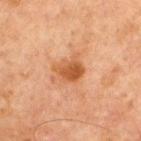Impression:
Part of a total-body skin-imaging series; this lesion was reviewed on a skin check and was not flagged for biopsy.
Acquisition and patient details:
The tile uses cross-polarized illumination. From the chest. Measured at roughly 3 mm in maximum diameter. Cropped from a total-body skin-imaging series; the visible field is about 15 mm. The patient is a male approximately 65 years of age.